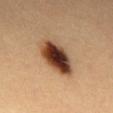The lesion was photographed on a routine skin check and not biopsied; there is no pathology result.
A male patient, aged approximately 20.
The lesion is on the mid back.
A region of skin cropped from a whole-body photographic capture, roughly 15 mm wide.
Captured under cross-polarized illumination.
The total-body-photography lesion software estimated an area of roughly 15 mm², a shape eccentricity near 0.8, and a symmetry-axis asymmetry near 0.15.